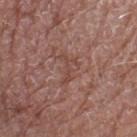Impression: Recorded during total-body skin imaging; not selected for excision or biopsy. Clinical summary: This image is a 15 mm lesion crop taken from a total-body photograph. The lesion is on the left lower leg. A male subject approximately 60 years of age.